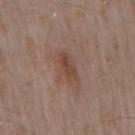| field | value |
|---|---|
| imaging modality | ~15 mm crop, total-body skin-cancer survey |
| patient | male, approximately 55 years of age |
| site | the arm |
| lighting | white-light |